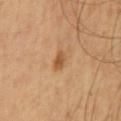Captured during whole-body skin photography for melanoma surveillance; the lesion was not biopsied.